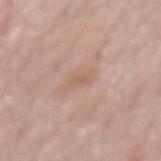Findings:
– subject · male, about 70 years old
– image · 15 mm crop, total-body photography
– anatomic site · the mid back